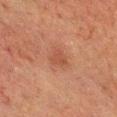{"biopsy_status": "not biopsied; imaged during a skin examination", "automated_metrics": {"area_mm2_approx": 3.5, "eccentricity": 0.7, "shape_asymmetry": 0.35, "border_irregularity_0_10": 3.0, "color_variation_0_10": 1.0, "peripheral_color_asymmetry": 0.5, "nevus_likeness_0_100": 20, "lesion_detection_confidence_0_100": 100}, "image": {"source": "total-body photography crop", "field_of_view_mm": 15}, "lighting": "cross-polarized", "site": "head or neck", "patient": {"sex": "male", "age_approx": 60}, "lesion_size": {"long_diameter_mm_approx": 2.5}}